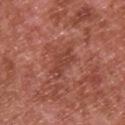The lesion's longest dimension is about 4 mm. The subject is a male roughly 65 years of age. The lesion is on the upper back. Cropped from a whole-body photographic skin survey; the tile spans about 15 mm.